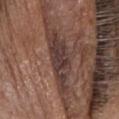This lesion was catalogued during total-body skin photography and was not selected for biopsy.
From the head or neck.
Captured under white-light illumination.
The subject is a female in their mid- to late 50s.
A 15 mm close-up extracted from a 3D total-body photography capture.
The lesion-visualizer software estimated a lesion area of about 14 mm², an eccentricity of roughly 0.95, and a shape-asymmetry score of about 0.3 (0 = symmetric). The software also gave a lesion color around L≈37 a*≈16 b*≈20 in CIELAB, roughly 11 lightness units darker than nearby skin, and a lesion-to-skin contrast of about 10 (normalized; higher = more distinct). The analysis additionally found an automated nevus-likeness rating near 0 out of 100 and a detector confidence of about 0 out of 100 that the crop contains a lesion.